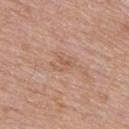Part of a total-body skin-imaging series; this lesion was reviewed on a skin check and was not flagged for biopsy.
A region of skin cropped from a whole-body photographic capture, roughly 15 mm wide.
From the upper back.
A male subject, roughly 75 years of age.